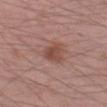Impression:
Captured during whole-body skin photography for melanoma surveillance; the lesion was not biopsied.
Image and clinical context:
Cropped from a total-body skin-imaging series; the visible field is about 15 mm. Imaged with white-light lighting. An algorithmic analysis of the crop reported an area of roughly 5.5 mm² and an outline eccentricity of about 0.3 (0 = round, 1 = elongated). And it measured a border-irregularity rating of about 2.5/10, internal color variation of about 3 on a 0–10 scale, and radial color variation of about 1. The software also gave a classifier nevus-likeness of about 35/100 and a detector confidence of about 100 out of 100 that the crop contains a lesion. The subject is a male aged 53–57. Longest diameter approximately 3 mm. The lesion is on the left thigh.